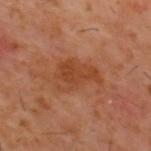Impression: No biopsy was performed on this lesion — it was imaged during a full skin examination and was not determined to be concerning. Background: Located on the back. A male patient about 60 years old. Captured under cross-polarized illumination. Measured at roughly 5 mm in maximum diameter. A 15 mm close-up tile from a total-body photography series done for melanoma screening. Automated tile analysis of the lesion measured border irregularity of about 4.5 on a 0–10 scale and internal color variation of about 3 on a 0–10 scale.